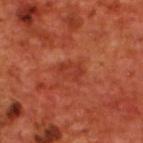<lesion>
<biopsy_status>not biopsied; imaged during a skin examination</biopsy_status>
<site>upper back</site>
<patient>
  <sex>male</sex>
  <age_approx>70</age_approx>
</patient>
<automated_metrics>
  <vs_skin_darker_L>6.0</vs_skin_darker_L>
  <vs_skin_contrast_norm>5.0</vs_skin_contrast_norm>
  <border_irregularity_0_10>2.5</border_irregularity_0_10>
  <color_variation_0_10>3.0</color_variation_0_10>
  <peripheral_color_asymmetry>1.0</peripheral_color_asymmetry>
</automated_metrics>
<image>
  <source>total-body photography crop</source>
  <field_of_view_mm>15</field_of_view_mm>
</image>
<lesion_size>
  <long_diameter_mm_approx>3.0</long_diameter_mm_approx>
</lesion_size>
<lighting>cross-polarized</lighting>
</lesion>